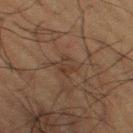Q: Is there a histopathology result?
A: no biopsy performed (imaged during a skin exam)
Q: Patient demographics?
A: male, aged approximately 60
Q: What lighting was used for the tile?
A: cross-polarized
Q: What is the imaging modality?
A: total-body-photography crop, ~15 mm field of view
Q: How large is the lesion?
A: ≈2.5 mm
Q: Where on the body is the lesion?
A: the right thigh